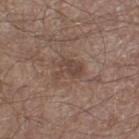TBP lesion metrics = an area of roughly 6 mm², an eccentricity of roughly 0.7, and a symmetry-axis asymmetry near 0.4; border irregularity of about 5 on a 0–10 scale and internal color variation of about 2.5 on a 0–10 scale; a nevus-likeness score of about 0/100 and a detector confidence of about 100 out of 100 that the crop contains a lesion | subject = male, roughly 55 years of age | acquisition = 15 mm crop, total-body photography | diameter = ~3.5 mm (longest diameter) | anatomic site = the left thigh | illumination = white-light illumination.